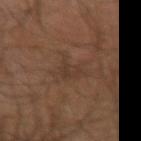Captured during whole-body skin photography for melanoma surveillance; the lesion was not biopsied. Located on the right upper arm. This image is a 15 mm lesion crop taken from a total-body photograph. A male subject, in their mid-60s. An algorithmic analysis of the crop reported an area of roughly 4 mm², a shape eccentricity near 0.7, and two-axis asymmetry of about 0.5. The software also gave a lesion color around L≈33 a*≈15 b*≈24 in CIELAB, roughly 5 lightness units darker than nearby skin, and a normalized lesion–skin contrast near 4.5. And it measured a nevus-likeness score of about 0/100 and a detector confidence of about 90 out of 100 that the crop contains a lesion. The lesion's longest dimension is about 3 mm.